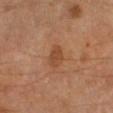Q: Is there a histopathology result?
A: no biopsy performed (imaged during a skin exam)
Q: What is the imaging modality?
A: ~15 mm tile from a whole-body skin photo
Q: Lesion location?
A: the left lower leg
Q: Lesion size?
A: about 3 mm
Q: Patient demographics?
A: male, roughly 65 years of age